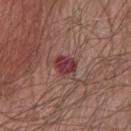The lesion was tiled from a total-body skin photograph and was not biopsied.
A close-up tile cropped from a whole-body skin photograph, about 15 mm across.
A male subject, aged 63 to 67.
An algorithmic analysis of the crop reported a footprint of about 5 mm². The analysis additionally found a detector confidence of about 100 out of 100 that the crop contains a lesion.
Imaged with white-light lighting.
Measured at roughly 3 mm in maximum diameter.
Located on the chest.